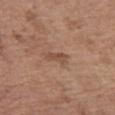Clinical impression:
Part of a total-body skin-imaging series; this lesion was reviewed on a skin check and was not flagged for biopsy.
Context:
The lesion's longest dimension is about 3 mm. The patient is a female aged approximately 65. The lesion is on the leg. This is a white-light tile. A region of skin cropped from a whole-body photographic capture, roughly 15 mm wide. The lesion-visualizer software estimated a lesion area of about 3 mm² and a shape eccentricity near 0.9. It also reported an average lesion color of about L≈49 a*≈20 b*≈29 (CIELAB), a lesion–skin lightness drop of about 8, and a lesion-to-skin contrast of about 6.5 (normalized; higher = more distinct). And it measured border irregularity of about 4 on a 0–10 scale, a within-lesion color-variation index near 0/10, and radial color variation of about 0.